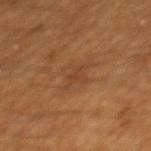The lesion was tiled from a total-body skin photograph and was not biopsied.
Approximately 2.5 mm at its widest.
This image is a 15 mm lesion crop taken from a total-body photograph.
The tile uses cross-polarized illumination.
A male subject, aged approximately 60.
On the mid back.
An algorithmic analysis of the crop reported a mean CIELAB color near L≈38 a*≈20 b*≈31, a lesion–skin lightness drop of about 5, and a normalized border contrast of about 4.5. The software also gave border irregularity of about 4.5 on a 0–10 scale, a color-variation rating of about 1.5/10, and a peripheral color-asymmetry measure near 0.5. The software also gave a nevus-likeness score of about 0/100.